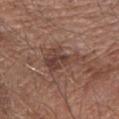follow-up — catalogued during a skin exam; not biopsied.